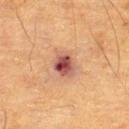Case summary:
• site — the right thigh
• imaging modality — ~15 mm tile from a whole-body skin photo
• illumination — cross-polarized
• lesion diameter — ≈3.5 mm
• image-analysis metrics — a footprint of about 7.5 mm², a shape eccentricity near 0.6, and two-axis asymmetry of about 0.15; a border-irregularity index near 1.5/10, a within-lesion color-variation index near 8.5/10, and a peripheral color-asymmetry measure near 2.5; a lesion-detection confidence of about 100/100
• subject — male, roughly 85 years of age or older